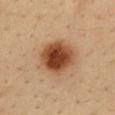biopsy_status: not biopsied; imaged during a skin examination
lesion_size:
  long_diameter_mm_approx: 5.0
automated_metrics:
  border_irregularity_0_10: 1.5
  peripheral_color_asymmetry: 1.5
site: back
image:
  source: total-body photography crop
  field_of_view_mm: 15
lighting: cross-polarized
patient:
  sex: male
  age_approx: 35